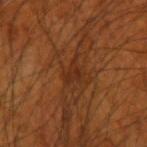Captured during whole-body skin photography for melanoma surveillance; the lesion was not biopsied. Located on the left forearm. A region of skin cropped from a whole-body photographic capture, roughly 15 mm wide. The patient is a male aged around 70.